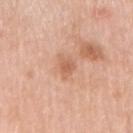{"biopsy_status": "not biopsied; imaged during a skin examination", "image": {"source": "total-body photography crop", "field_of_view_mm": 15}, "patient": {"sex": "male", "age_approx": 80}, "site": "right upper arm", "lesion_size": {"long_diameter_mm_approx": 3.0}, "lighting": "white-light"}